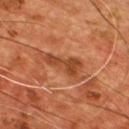The lesion was photographed on a routine skin check and not biopsied; there is no pathology result.
The subject is a male roughly 55 years of age.
Cropped from a total-body skin-imaging series; the visible field is about 15 mm.
On the chest.